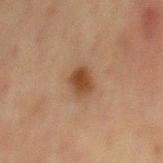Image and clinical context:
About 3 mm across. A lesion tile, about 15 mm wide, cut from a 3D total-body photograph. Captured under cross-polarized illumination. The patient is a male roughly 65 years of age. Automated image analysis of the tile measured a lesion-detection confidence of about 100/100. From the front of the torso.